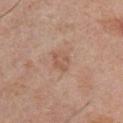– notes · total-body-photography surveillance lesion; no biopsy
– image source · ~15 mm crop, total-body skin-cancer survey
– lighting · white-light illumination
– patient · male, approximately 30 years of age
– anatomic site · the chest
– TBP lesion metrics · a footprint of about 4.5 mm², an eccentricity of roughly 0.5, and a shape-asymmetry score of about 0.25 (0 = symmetric); an average lesion color of about L≈56 a*≈20 b*≈30 (CIELAB), about 7 CIELAB-L* units darker than the surrounding skin, and a normalized border contrast of about 5; an automated nevus-likeness rating near 0 out of 100 and lesion-presence confidence of about 100/100
– size · about 2.5 mm